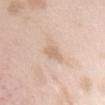The lesion was tiled from a total-body skin photograph and was not biopsied. This is a white-light tile. About 3 mm across. On the chest. Cropped from a whole-body photographic skin survey; the tile spans about 15 mm. An algorithmic analysis of the crop reported an area of roughly 3 mm². The analysis additionally found a lesion color around L≈68 a*≈15 b*≈30 in CIELAB and a normalized border contrast of about 5.5. And it measured border irregularity of about 3.5 on a 0–10 scale and peripheral color asymmetry of about 0. And it measured an automated nevus-likeness rating near 0 out of 100 and a lesion-detection confidence of about 100/100. A female patient in their mid- to late 20s.